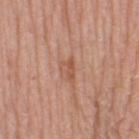Clinical impression: Imaged during a routine full-body skin examination; the lesion was not biopsied and no histopathology is available. Image and clinical context: A roughly 15 mm field-of-view crop from a total-body skin photograph. The lesion is located on the leg. A female subject in their mid- to late 60s. The tile uses white-light illumination. The lesion's longest dimension is about 2.5 mm.